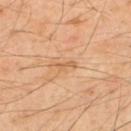No biopsy was performed on this lesion — it was imaged during a full skin examination and was not determined to be concerning. A male subject in their 50s. A roughly 15 mm field-of-view crop from a total-body skin photograph. Captured under cross-polarized illumination. The lesion is located on the upper back.